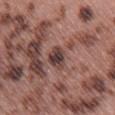biopsy_status: not biopsied; imaged during a skin examination
automated_metrics:
  eccentricity: 0.75
  cielab_L: 40
  cielab_a: 18
  cielab_b: 20
  vs_skin_darker_L: 12.0
  border_irregularity_0_10: 2.5
  color_variation_0_10: 5.0
  peripheral_color_asymmetry: 1.5
site: right thigh
patient:
  sex: female
  age_approx: 60
image:
  source: total-body photography crop
  field_of_view_mm: 15